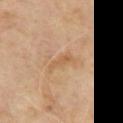{"biopsy_status": "not biopsied; imaged during a skin examination", "lesion_size": {"long_diameter_mm_approx": 3.0}, "image": {"source": "total-body photography crop", "field_of_view_mm": 15}, "patient": {"sex": "male", "age_approx": 70}, "automated_metrics": {"cielab_L": 57, "cielab_a": 19, "cielab_b": 36, "vs_skin_contrast_norm": 5.5, "nevus_likeness_0_100": 0, "lesion_detection_confidence_0_100": 100}, "lighting": "cross-polarized", "site": "chest"}